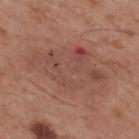notes = catalogued during a skin exam; not biopsied | diameter = about 9.5 mm | patient = male, aged approximately 55 | tile lighting = white-light | anatomic site = the upper back | acquisition = total-body-photography crop, ~15 mm field of view.